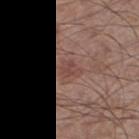Findings:
* image — ~15 mm crop, total-body skin-cancer survey
* location — the right thigh
* patient — male, in their 60s